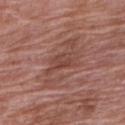Findings:
– workup — imaged on a skin check; not biopsied
– subject — female, aged around 70
– location — the right upper arm
– lighting — white-light illumination
– image — total-body-photography crop, ~15 mm field of view
– TBP lesion metrics — a lesion–skin lightness drop of about 7; a border-irregularity index near 5/10, a color-variation rating of about 2.5/10, and radial color variation of about 1; an automated nevus-likeness rating near 0 out of 100 and a lesion-detection confidence of about 95/100